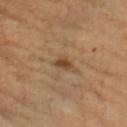tile lighting — cross-polarized | site — the arm | acquisition — ~15 mm crop, total-body skin-cancer survey | image-analysis metrics — a border-irregularity index near 3.5/10, a color-variation rating of about 2/10, and radial color variation of about 0.5 | subject — female, in their 60s | lesion size — ≈2.5 mm.